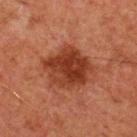Q: Is there a histopathology result?
A: total-body-photography surveillance lesion; no biopsy
Q: Lesion size?
A: about 5.5 mm
Q: What is the anatomic site?
A: the chest
Q: Patient demographics?
A: male, aged approximately 60
Q: What did automated image analysis measure?
A: an outline eccentricity of about 0.5 (0 = round, 1 = elongated) and two-axis asymmetry of about 0.2; a classifier nevus-likeness of about 90/100 and a detector confidence of about 100 out of 100 that the crop contains a lesion
Q: What kind of image is this?
A: total-body-photography crop, ~15 mm field of view
Q: How was the tile lit?
A: cross-polarized